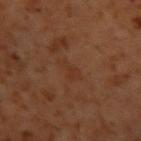This lesion was catalogued during total-body skin photography and was not selected for biopsy.
The recorded lesion diameter is about 3.5 mm.
A lesion tile, about 15 mm wide, cut from a 3D total-body photograph.
A male patient aged 58–62.
Located on the left upper arm.
Captured under cross-polarized illumination.
Automated image analysis of the tile measured internal color variation of about 0.5 on a 0–10 scale and a peripheral color-asymmetry measure near 0.